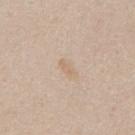A 15 mm close-up extracted from a 3D total-body photography capture. Longest diameter approximately 2.5 mm. A female subject, approximately 45 years of age. This is a white-light tile. Located on the mid back.